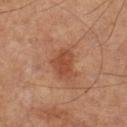Part of a total-body skin-imaging series; this lesion was reviewed on a skin check and was not flagged for biopsy. The lesion-visualizer software estimated a footprint of about 7.5 mm², an outline eccentricity of about 0.75 (0 = round, 1 = elongated), and a shape-asymmetry score of about 0.35 (0 = symmetric). It also reported a detector confidence of about 100 out of 100 that the crop contains a lesion. On the left lower leg. A roughly 15 mm field-of-view crop from a total-body skin photograph. Captured under cross-polarized illumination. Longest diameter approximately 4 mm. The subject is a male aged 63 to 67.